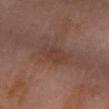workup: no biopsy performed (imaged during a skin exam)
subject: male, roughly 55 years of age
size: ≈4 mm
image source: total-body-photography crop, ~15 mm field of view
anatomic site: the left forearm